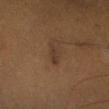  biopsy_status: not biopsied; imaged during a skin examination
  image:
    source: total-body photography crop
    field_of_view_mm: 15
  site: right lower leg
  automated_metrics:
    vs_skin_darker_L: 5.0
    vs_skin_contrast_norm: 6.0
    border_irregularity_0_10: 3.5
    color_variation_0_10: 1.0
    peripheral_color_asymmetry: 0.0
  patient:
    sex: male
    age_approx: 60